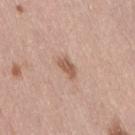Acquisition and patient details: A roughly 15 mm field-of-view crop from a total-body skin photograph. The lesion is on the lower back. The lesion-visualizer software estimated an average lesion color of about L≈57 a*≈20 b*≈29 (CIELAB) and a normalized lesion–skin contrast near 7.5. The analysis additionally found lesion-presence confidence of about 100/100. A female patient, aged approximately 40.